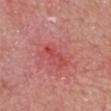This lesion was catalogued during total-body skin photography and was not selected for biopsy. This is a white-light tile. This image is a 15 mm lesion crop taken from a total-body photograph. The patient is a male aged approximately 80. On the head or neck.